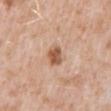The lesion was tiled from a total-body skin photograph and was not biopsied. A male patient in their 50s. A lesion tile, about 15 mm wide, cut from a 3D total-body photograph. The lesion is located on the mid back. The total-body-photography lesion software estimated border irregularity of about 1.5 on a 0–10 scale, internal color variation of about 4 on a 0–10 scale, and a peripheral color-asymmetry measure near 1.5. And it measured a classifier nevus-likeness of about 85/100 and lesion-presence confidence of about 100/100. The tile uses white-light illumination. Measured at roughly 2.5 mm in maximum diameter.